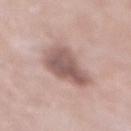Imaged with white-light lighting. The lesion is on the abdomen. Measured at roughly 6 mm in maximum diameter. A male subject aged 53–57. Cropped from a total-body skin-imaging series; the visible field is about 15 mm.